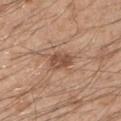Recorded during total-body skin imaging; not selected for excision or biopsy. A close-up tile cropped from a whole-body skin photograph, about 15 mm across. Imaged with white-light lighting. Measured at roughly 2.5 mm in maximum diameter. The lesion is on the arm. The subject is a male aged approximately 50. The total-body-photography lesion software estimated a lesion color around L≈49 a*≈20 b*≈29 in CIELAB, a lesion–skin lightness drop of about 11, and a normalized lesion–skin contrast near 8. It also reported internal color variation of about 3 on a 0–10 scale and peripheral color asymmetry of about 1.5. The software also gave an automated nevus-likeness rating near 25 out of 100.